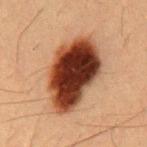The lesion was tiled from a total-body skin photograph and was not biopsied. The lesion is on the chest. Longest diameter approximately 8.5 mm. Captured under cross-polarized illumination. The subject is a male aged approximately 55. A 15 mm crop from a total-body photograph taken for skin-cancer surveillance.